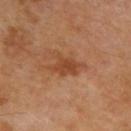- notes · imaged on a skin check; not biopsied
- location · the upper back
- image source · ~15 mm crop, total-body skin-cancer survey
- illumination · cross-polarized illumination
- lesion size · ~4 mm (longest diameter)
- patient · male, aged 68–72